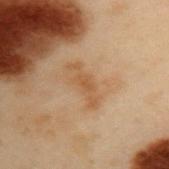  biopsy_status: not biopsied; imaged during a skin examination
  patient:
    sex: male
    age_approx: 55
  site: upper back
  image:
    source: total-body photography crop
    field_of_view_mm: 15